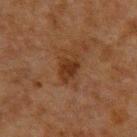follow-up — total-body-photography surveillance lesion; no biopsy | acquisition — ~15 mm tile from a whole-body skin photo | subject — male, aged 48–52 | site — the upper back | tile lighting — cross-polarized illumination | size — about 3.5 mm.